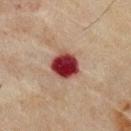patient:
  sex: male
  age_approx: 70
image:
  source: total-body photography crop
  field_of_view_mm: 15
site: chest
lighting: cross-polarized